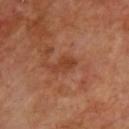The lesion was tiled from a total-body skin photograph and was not biopsied. A male subject aged approximately 65. A 15 mm crop from a total-body photograph taken for skin-cancer surveillance. The lesion's longest dimension is about 3 mm. Automated image analysis of the tile measured a lesion color around L≈40 a*≈25 b*≈33 in CIELAB, roughly 7 lightness units darker than nearby skin, and a normalized border contrast of about 6. The analysis additionally found a border-irregularity index near 2.5/10, a color-variation rating of about 1.5/10, and peripheral color asymmetry of about 0.5. The software also gave a nevus-likeness score of about 0/100 and lesion-presence confidence of about 100/100. The tile uses cross-polarized illumination.